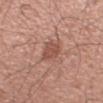Impression:
No biopsy was performed on this lesion — it was imaged during a full skin examination and was not determined to be concerning.
Clinical summary:
Longest diameter approximately 2.5 mm. A lesion tile, about 15 mm wide, cut from a 3D total-body photograph. A male patient aged around 35. The lesion-visualizer software estimated a lesion area of about 5.5 mm² and an eccentricity of roughly 0.2. It also reported border irregularity of about 2 on a 0–10 scale, a color-variation rating of about 2/10, and peripheral color asymmetry of about 0.5. From the left upper arm.